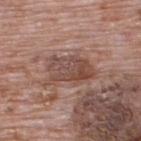Q: Is there a histopathology result?
A: catalogued during a skin exam; not biopsied
Q: What are the patient's age and sex?
A: male, approximately 70 years of age
Q: How was the tile lit?
A: white-light illumination
Q: What did automated image analysis measure?
A: a footprint of about 13 mm², an outline eccentricity of about 0.8 (0 = round, 1 = elongated), and two-axis asymmetry of about 0.25
Q: How was this image acquired?
A: 15 mm crop, total-body photography
Q: What is the anatomic site?
A: the upper back